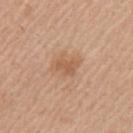<case>
  <biopsy_status>not biopsied; imaged during a skin examination</biopsy_status>
  <site>left upper arm</site>
  <lighting>white-light</lighting>
  <automated_metrics>
    <border_irregularity_0_10>3.5</border_irregularity_0_10>
    <color_variation_0_10>2.0</color_variation_0_10>
    <peripheral_color_asymmetry>0.5</peripheral_color_asymmetry>
  </automated_metrics>
  <patient>
    <sex>female</sex>
    <age_approx>60</age_approx>
  </patient>
  <image>
    <source>total-body photography crop</source>
    <field_of_view_mm>15</field_of_view_mm>
  </image>
  <lesion_size>
    <long_diameter_mm_approx>3.0</long_diameter_mm_approx>
  </lesion_size>
</case>